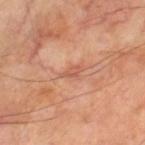notes = no biopsy performed (imaged during a skin exam)
image source = ~15 mm tile from a whole-body skin photo
patient = male, in their 70s
location = the leg